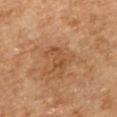notes: catalogued during a skin exam; not biopsied | lesion size: about 3.5 mm | site: the upper back | lighting: cross-polarized illumination | image: ~15 mm tile from a whole-body skin photo | patient: female, aged around 60 | automated metrics: a footprint of about 4.5 mm², an outline eccentricity of about 0.9 (0 = round, 1 = elongated), and a shape-asymmetry score of about 0.4 (0 = symmetric); an average lesion color of about L≈46 a*≈21 b*≈34 (CIELAB) and a lesion-to-skin contrast of about 5.5 (normalized; higher = more distinct).